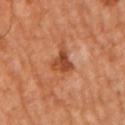| feature | finding |
|---|---|
| follow-up | no biopsy performed (imaged during a skin exam) |
| image | 15 mm crop, total-body photography |
| size | ≈3.5 mm |
| site | the right upper arm |
| subject | male, in their mid- to late 60s |
| tile lighting | cross-polarized |